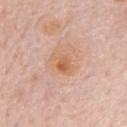Q: Was a biopsy performed?
A: no biopsy performed (imaged during a skin exam)
Q: What is the anatomic site?
A: the chest
Q: How was this image acquired?
A: total-body-photography crop, ~15 mm field of view
Q: What lighting was used for the tile?
A: white-light illumination
Q: Patient demographics?
A: male, aged 78–82